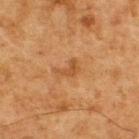No biopsy was performed on this lesion — it was imaged during a full skin examination and was not determined to be concerning. The lesion is on the back. The patient is a male aged approximately 75. The total-body-photography lesion software estimated a lesion area of about 3 mm², an outline eccentricity of about 0.8 (0 = round, 1 = elongated), and a symmetry-axis asymmetry near 0.6. And it measured a lesion color around L≈41 a*≈20 b*≈34 in CIELAB, a lesion–skin lightness drop of about 7, and a normalized border contrast of about 6. And it measured a nevus-likeness score of about 0/100 and a detector confidence of about 100 out of 100 that the crop contains a lesion. About 3 mm across. The tile uses cross-polarized illumination. A lesion tile, about 15 mm wide, cut from a 3D total-body photograph.